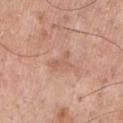biopsy status = catalogued during a skin exam; not biopsied